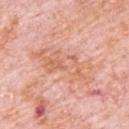  biopsy_status: not biopsied; imaged during a skin examination
  site: upper back
  lesion_size:
    long_diameter_mm_approx: 8.0
  image:
    source: total-body photography crop
    field_of_view_mm: 15
  lighting: white-light
  patient:
    sex: male
    age_approx: 80
  automated_metrics:
    area_mm2_approx: 17.0
    eccentricity: 0.95
    shape_asymmetry: 0.4
    vs_skin_darker_L: 7.0
    vs_skin_contrast_norm: 5.5
    color_variation_0_10: 5.0
    peripheral_color_asymmetry: 1.5